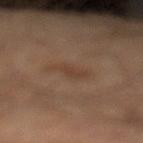Assessment:
This lesion was catalogued during total-body skin photography and was not selected for biopsy.
Image and clinical context:
Automated image analysis of the tile measured a footprint of about 4 mm², a shape eccentricity near 0.9, and a shape-asymmetry score of about 0.35 (0 = symmetric). The recorded lesion diameter is about 3.5 mm. The tile uses cross-polarized illumination. On the left leg. The patient is a male aged 48 to 52. This image is a 15 mm lesion crop taken from a total-body photograph.